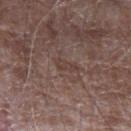Part of a total-body skin-imaging series; this lesion was reviewed on a skin check and was not flagged for biopsy. A roughly 15 mm field-of-view crop from a total-body skin photograph. From the left forearm. The subject is a male approximately 65 years of age.